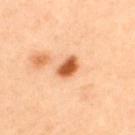workup: no biopsy performed (imaged during a skin exam)
diameter: ≈3 mm
site: the upper back
automated lesion analysis: a lesion area of about 5 mm², an eccentricity of roughly 0.8, and a shape-asymmetry score of about 0.25 (0 = symmetric); a lesion color around L≈59 a*≈29 b*≈42 in CIELAB; border irregularity of about 2.5 on a 0–10 scale and a peripheral color-asymmetry measure near 1
image: ~15 mm tile from a whole-body skin photo
lighting: cross-polarized illumination
subject: male, aged approximately 45